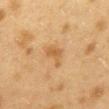<lesion>
  <biopsy_status>not biopsied; imaged during a skin examination</biopsy_status>
  <image>
    <source>total-body photography crop</source>
    <field_of_view_mm>15</field_of_view_mm>
  </image>
  <lighting>cross-polarized</lighting>
  <patient>
    <sex>female</sex>
    <age_approx>40</age_approx>
  </patient>
  <site>mid back</site>
</lesion>